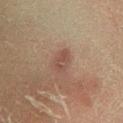Captured during whole-body skin photography for melanoma surveillance; the lesion was not biopsied. The tile uses cross-polarized illumination. On the left lower leg. The lesion's longest dimension is about 3 mm. A male subject, aged 58–62. A 15 mm close-up tile from a total-body photography series done for melanoma screening.